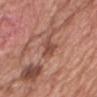Notes:
* follow-up: total-body-photography surveillance lesion; no biopsy
* patient: male, aged approximately 60
* illumination: white-light
* acquisition: ~15 mm crop, total-body skin-cancer survey
* size: ≈3.5 mm
* location: the chest
* automated lesion analysis: a lesion area of about 5.5 mm² and an eccentricity of roughly 0.8; a border-irregularity index near 4/10